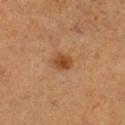follow-up=imaged on a skin check; not biopsied
body site=the left leg
patient=female, aged around 45
automated lesion analysis=border irregularity of about 2.5 on a 0–10 scale, a within-lesion color-variation index near 3.5/10, and peripheral color asymmetry of about 1; a detector confidence of about 100 out of 100 that the crop contains a lesion
lesion diameter=≈2.5 mm
image=total-body-photography crop, ~15 mm field of view